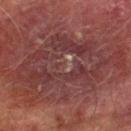Clinical summary:
A male patient, approximately 75 years of age. Captured under cross-polarized illumination. The recorded lesion diameter is about 12.5 mm. The lesion is located on the right lower leg. A 15 mm crop from a total-body photograph taken for skin-cancer surveillance.